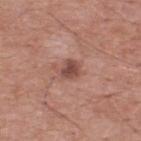Assessment:
Part of a total-body skin-imaging series; this lesion was reviewed on a skin check and was not flagged for biopsy.
Clinical summary:
The lesion-visualizer software estimated a footprint of about 5 mm², an outline eccentricity of about 0.65 (0 = round, 1 = elongated), and two-axis asymmetry of about 0.3. The software also gave a mean CIELAB color near L≈48 a*≈22 b*≈25 and a normalized lesion–skin contrast near 8. And it measured border irregularity of about 2.5 on a 0–10 scale and internal color variation of about 3 on a 0–10 scale. And it measured a classifier nevus-likeness of about 55/100 and a detector confidence of about 100 out of 100 that the crop contains a lesion. A male subject, roughly 55 years of age. Cropped from a total-body skin-imaging series; the visible field is about 15 mm. About 3 mm across. Captured under white-light illumination. The lesion is located on the back.